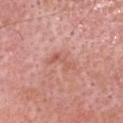Part of a total-body skin-imaging series; this lesion was reviewed on a skin check and was not flagged for biopsy. A 15 mm close-up extracted from a 3D total-body photography capture. Captured under white-light illumination. A male subject in their 50s. Longest diameter approximately 4 mm. The total-body-photography lesion software estimated a footprint of about 4.5 mm², an outline eccentricity of about 0.9 (0 = round, 1 = elongated), and a shape-asymmetry score of about 0.5 (0 = symmetric). And it measured a lesion-detection confidence of about 100/100. On the head or neck.